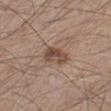Part of a total-body skin-imaging series; this lesion was reviewed on a skin check and was not flagged for biopsy.
Automated image analysis of the tile measured a footprint of about 6.5 mm², an outline eccentricity of about 0.85 (0 = round, 1 = elongated), and two-axis asymmetry of about 0.25. The analysis additionally found peripheral color asymmetry of about 1.5. And it measured a classifier nevus-likeness of about 85/100 and lesion-presence confidence of about 100/100.
Imaged with white-light lighting.
On the left lower leg.
Measured at roughly 4 mm in maximum diameter.
A 15 mm crop from a total-body photograph taken for skin-cancer surveillance.
The patient is a male in their mid- to late 40s.